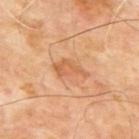| key | value |
|---|---|
| follow-up | imaged on a skin check; not biopsied |
| patient | male, aged 63 to 67 |
| anatomic site | the upper back |
| acquisition | 15 mm crop, total-body photography |